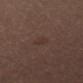Q: Was this lesion biopsied?
A: catalogued during a skin exam; not biopsied
Q: Lesion location?
A: the right thigh
Q: Patient demographics?
A: female, about 45 years old
Q: What lighting was used for the tile?
A: cross-polarized
Q: What did automated image analysis measure?
A: a shape eccentricity near 0.8 and a shape-asymmetry score of about 0.35 (0 = symmetric); an average lesion color of about L≈31 a*≈15 b*≈19 (CIELAB), a lesion–skin lightness drop of about 4, and a lesion-to-skin contrast of about 4 (normalized; higher = more distinct); an automated nevus-likeness rating near 0 out of 100 and a lesion-detection confidence of about 100/100
Q: What is the imaging modality?
A: 15 mm crop, total-body photography
Q: How large is the lesion?
A: about 2.5 mm